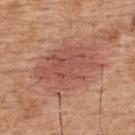{
  "biopsy_status": "not biopsied; imaged during a skin examination",
  "site": "upper back",
  "patient": {
    "sex": "male",
    "age_approx": 60
  },
  "lighting": "white-light",
  "automated_metrics": {
    "color_variation_0_10": 4.0,
    "peripheral_color_asymmetry": 1.5
  },
  "image": {
    "source": "total-body photography crop",
    "field_of_view_mm": 15
  }
}